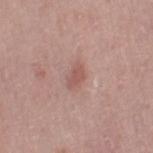Part of a total-body skin-imaging series; this lesion was reviewed on a skin check and was not flagged for biopsy. The subject is a female aged 48–52. The lesion is located on the right thigh. A 15 mm crop from a total-body photograph taken for skin-cancer surveillance. The recorded lesion diameter is about 2.5 mm. An algorithmic analysis of the crop reported border irregularity of about 2.5 on a 0–10 scale, a within-lesion color-variation index near 1/10, and peripheral color asymmetry of about 0.5.